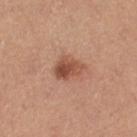Notes:
* biopsy status: catalogued during a skin exam; not biopsied
* lesion size: about 3.5 mm
* body site: the left thigh
* image-analysis metrics: a shape eccentricity near 0.75 and two-axis asymmetry of about 0.3; a nevus-likeness score of about 90/100 and a detector confidence of about 100 out of 100 that the crop contains a lesion
* illumination: white-light
* subject: female, aged approximately 45
* image source: 15 mm crop, total-body photography